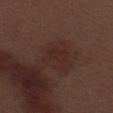Part of a total-body skin-imaging series; this lesion was reviewed on a skin check and was not flagged for biopsy. The lesion is located on the left thigh. A male subject, roughly 70 years of age. A roughly 15 mm field-of-view crop from a total-body skin photograph. Imaged with white-light lighting. About 3.5 mm across.